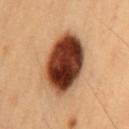patient:
  sex: male
  age_approx: 55
lesion_size:
  long_diameter_mm_approx: 7.0
image:
  source: total-body photography crop
  field_of_view_mm: 15
automated_metrics:
  area_mm2_approx: 29.0
  eccentricity: 0.65
  vs_skin_darker_L: 23.0
  vs_skin_contrast_norm: 18.0
site: lower back